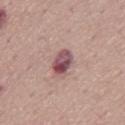biopsy status=no biopsy performed (imaged during a skin exam)
subject=male, aged around 70
lighting=white-light
anatomic site=the mid back
automated metrics=a footprint of about 6.5 mm²; a border-irregularity rating of about 2/10 and peripheral color asymmetry of about 3.5
acquisition=total-body-photography crop, ~15 mm field of view
lesion size=about 4 mm